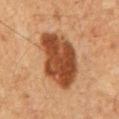biopsy status = no biopsy performed (imaged during a skin exam).